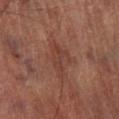- biopsy status — catalogued during a skin exam; not biopsied
- acquisition — ~15 mm crop, total-body skin-cancer survey
- subject — male, aged around 70
- location — the right lower leg
- diameter — ≈5 mm